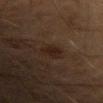Clinical impression: Recorded during total-body skin imaging; not selected for excision or biopsy. Context: The recorded lesion diameter is about 2.5 mm. Cropped from a whole-body photographic skin survey; the tile spans about 15 mm. Imaged with cross-polarized lighting. An algorithmic analysis of the crop reported a mean CIELAB color near L≈16 a*≈13 b*≈18, a lesion–skin lightness drop of about 5, and a normalized border contrast of about 8. The software also gave internal color variation of about 1 on a 0–10 scale and peripheral color asymmetry of about 0.5. The software also gave an automated nevus-likeness rating near 60 out of 100 and a detector confidence of about 100 out of 100 that the crop contains a lesion. On the right forearm. The patient is a male roughly 70 years of age.